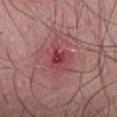Q: Was this lesion biopsied?
A: imaged on a skin check; not biopsied